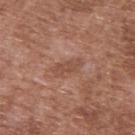| field | value |
|---|---|
| lesion size | ≈3.5 mm |
| TBP lesion metrics | an area of roughly 5 mm² and two-axis asymmetry of about 0.25; a border-irregularity index near 3/10 and a color-variation rating of about 2/10 |
| subject | male, in their mid- to late 70s |
| image | ~15 mm tile from a whole-body skin photo |
| lighting | white-light illumination |
| site | the upper back |